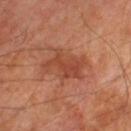Clinical impression:
The lesion was tiled from a total-body skin photograph and was not biopsied.
Image and clinical context:
The lesion-visualizer software estimated an eccentricity of roughly 0.8 and a shape-asymmetry score of about 0.4 (0 = symmetric). It also reported a border-irregularity index near 5.5/10 and a color-variation rating of about 3/10. The software also gave a classifier nevus-likeness of about 0/100 and lesion-presence confidence of about 100/100. A male patient aged approximately 70. A 15 mm crop from a total-body photograph taken for skin-cancer surveillance. From the right lower leg.